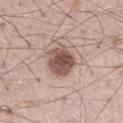Imaged during a routine full-body skin examination; the lesion was not biopsied and no histopathology is available. From the leg. A male subject, in their mid-50s. The recorded lesion diameter is about 4 mm. A roughly 15 mm field-of-view crop from a total-body skin photograph. Imaged with white-light lighting. An algorithmic analysis of the crop reported a footprint of about 11 mm² and a symmetry-axis asymmetry near 0.15.